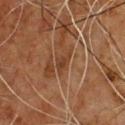Part of a total-body skin-imaging series; this lesion was reviewed on a skin check and was not flagged for biopsy.
Approximately 3 mm at its widest.
The patient is a male aged approximately 60.
The total-body-photography lesion software estimated an area of roughly 3.5 mm² and an eccentricity of roughly 0.85. And it measured a lesion color around L≈33 a*≈18 b*≈27 in CIELAB and a normalized lesion–skin contrast near 6.5. The software also gave border irregularity of about 4 on a 0–10 scale, internal color variation of about 1 on a 0–10 scale, and peripheral color asymmetry of about 0.5. The software also gave an automated nevus-likeness rating near 0 out of 100.
The lesion is on the chest.
A 15 mm crop from a total-body photograph taken for skin-cancer surveillance.